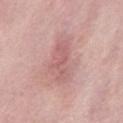Captured during whole-body skin photography for melanoma surveillance; the lesion was not biopsied. From the mid back. This is a white-light tile. A 15 mm close-up tile from a total-body photography series done for melanoma screening. Automated tile analysis of the lesion measured a footprint of about 15 mm², an eccentricity of roughly 0.85, and a shape-asymmetry score of about 0.25 (0 = symmetric). It also reported about 8 CIELAB-L* units darker than the surrounding skin. And it measured a border-irregularity index near 3/10, a within-lesion color-variation index near 3/10, and radial color variation of about 1. The subject is a male aged around 55.